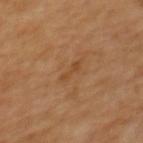Acquisition and patient details: A 15 mm crop from a total-body photograph taken for skin-cancer surveillance. On the upper back. The subject is a female in their mid- to late 60s.